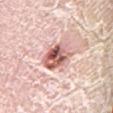workup: catalogued during a skin exam; not biopsied
site: the left lower leg
imaging modality: ~15 mm crop, total-body skin-cancer survey
subject: female, in their mid- to late 40s
tile lighting: white-light illumination